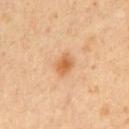Findings:
– follow-up · total-body-photography surveillance lesion; no biopsy